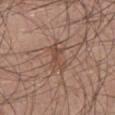Q: Was a biopsy performed?
A: total-body-photography surveillance lesion; no biopsy
Q: What kind of image is this?
A: ~15 mm tile from a whole-body skin photo
Q: Patient demographics?
A: male, roughly 30 years of age
Q: What is the anatomic site?
A: the leg
Q: How large is the lesion?
A: about 3 mm